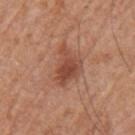| feature | finding |
|---|---|
| follow-up | catalogued during a skin exam; not biopsied |
| patient | male, about 65 years old |
| body site | the left upper arm |
| lesion size | about 5 mm |
| image | ~15 mm tile from a whole-body skin photo |